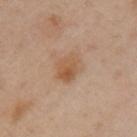Impression: The lesion was photographed on a routine skin check and not biopsied; there is no pathology result. Background: The lesion's longest dimension is about 3.5 mm. Cropped from a whole-body photographic skin survey; the tile spans about 15 mm. A female patient aged 38 to 42. This is a cross-polarized tile. The lesion is on the left upper arm. The total-body-photography lesion software estimated a lesion area of about 8 mm². It also reported a nevus-likeness score of about 25/100 and a detector confidence of about 100 out of 100 that the crop contains a lesion.